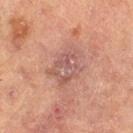{"biopsy_status": "not biopsied; imaged during a skin examination", "patient": {"sex": "male", "age_approx": 70}, "automated_metrics": {"border_irregularity_0_10": 3.5, "color_variation_0_10": 4.5, "nevus_likeness_0_100": 0, "lesion_detection_confidence_0_100": 95}, "lesion_size": {"long_diameter_mm_approx": 5.0}, "image": {"source": "total-body photography crop", "field_of_view_mm": 15}, "lighting": "cross-polarized", "site": "left thigh"}